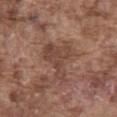Notes:
- acquisition: 15 mm crop, total-body photography
- automated lesion analysis: an area of roughly 12 mm² and an eccentricity of roughly 0.7; an average lesion color of about L≈44 a*≈19 b*≈25 (CIELAB), a lesion–skin lightness drop of about 8, and a lesion-to-skin contrast of about 6 (normalized; higher = more distinct); a within-lesion color-variation index near 3/10 and radial color variation of about 1; a nevus-likeness score of about 0/100
- anatomic site: the abdomen
- tile lighting: white-light
- patient: male, aged 73–77